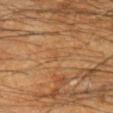| key | value |
|---|---|
| notes | total-body-photography surveillance lesion; no biopsy |
| image-analysis metrics | a border-irregularity rating of about 3/10, internal color variation of about 0 on a 0–10 scale, and radial color variation of about 0 |
| body site | the left forearm |
| imaging modality | ~15 mm crop, total-body skin-cancer survey |
| patient | male, in their 60s |